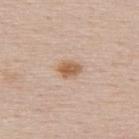A roughly 15 mm field-of-view crop from a total-body skin photograph. A male subject aged approximately 50. The lesion is on the back. Imaged with white-light lighting.